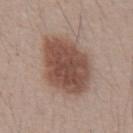| feature | finding |
|---|---|
| workup | total-body-photography surveillance lesion; no biopsy |
| illumination | white-light illumination |
| image | total-body-photography crop, ~15 mm field of view |
| diameter | ~6.5 mm (longest diameter) |
| body site | the abdomen |
| patient | male, approximately 40 years of age |